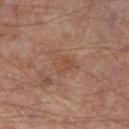No biopsy was performed on this lesion — it was imaged during a full skin examination and was not determined to be concerning.